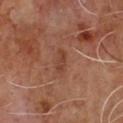Imaged during a routine full-body skin examination; the lesion was not biopsied and no histopathology is available. From the chest. A 15 mm close-up tile from a total-body photography series done for melanoma screening. The recorded lesion diameter is about 3 mm. This is a cross-polarized tile. The patient is a male roughly 65 years of age.